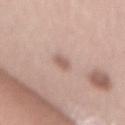No biopsy was performed on this lesion — it was imaged during a full skin examination and was not determined to be concerning.
A roughly 15 mm field-of-view crop from a total-body skin photograph.
A female patient, aged 63 to 67.
The lesion is on the mid back.
The total-body-photography lesion software estimated an area of roughly 3 mm², an eccentricity of roughly 0.85, and two-axis asymmetry of about 0.3. The software also gave an average lesion color of about L≈60 a*≈19 b*≈24 (CIELAB), roughly 9 lightness units darker than nearby skin, and a normalized lesion–skin contrast near 6. And it measured a within-lesion color-variation index near 2/10 and peripheral color asymmetry of about 0.5.
The lesion's longest dimension is about 2.5 mm.
The tile uses white-light illumination.